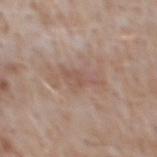<lesion>
  <biopsy_status>not biopsied; imaged during a skin examination</biopsy_status>
  <automated_metrics>
    <nevus_likeness_0_100>0</nevus_likeness_0_100>
    <lesion_detection_confidence_0_100>100</lesion_detection_confidence_0_100>
  </automated_metrics>
  <image>
    <source>total-body photography crop</source>
    <field_of_view_mm>15</field_of_view_mm>
  </image>
  <patient>
    <sex>male</sex>
    <age_approx>50</age_approx>
  </patient>
  <site>upper back</site>
  <lighting>white-light</lighting>
</lesion>